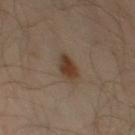| feature | finding |
|---|---|
| follow-up | total-body-photography surveillance lesion; no biopsy |
| image source | ~15 mm crop, total-body skin-cancer survey |
| subject | male, aged 38–42 |
| location | the left thigh |
| automated lesion analysis | a mean CIELAB color near L≈37 a*≈16 b*≈27, about 10 CIELAB-L* units darker than the surrounding skin, and a normalized lesion–skin contrast near 9.5; an automated nevus-likeness rating near 95 out of 100 and a lesion-detection confidence of about 100/100 |
| size | about 3 mm |